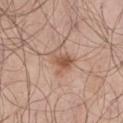Recorded during total-body skin imaging; not selected for excision or biopsy.
Imaged with white-light lighting.
A close-up tile cropped from a whole-body skin photograph, about 15 mm across.
On the leg.
About 3.5 mm across.
A male patient aged around 70.